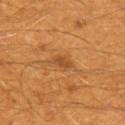Clinical impression: The lesion was tiled from a total-body skin photograph and was not biopsied. Acquisition and patient details: The lesion is on the upper back. A male patient, about 60 years old. This is a cross-polarized tile. Measured at roughly 2.5 mm in maximum diameter. A lesion tile, about 15 mm wide, cut from a 3D total-body photograph.